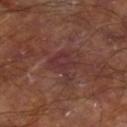Captured during whole-body skin photography for melanoma surveillance; the lesion was not biopsied. A male patient aged 58 to 62. Longest diameter approximately 3.5 mm. The total-body-photography lesion software estimated a footprint of about 7 mm², an eccentricity of roughly 0.55, and a shape-asymmetry score of about 0.5 (0 = symmetric). It also reported a border-irregularity rating of about 6/10, a color-variation rating of about 2.5/10, and radial color variation of about 1. The software also gave a nevus-likeness score of about 0/100 and lesion-presence confidence of about 85/100. This is a cross-polarized tile. The lesion is on the right lower leg. Cropped from a whole-body photographic skin survey; the tile spans about 15 mm.